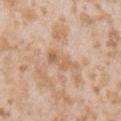This lesion was catalogued during total-body skin photography and was not selected for biopsy. This is a white-light tile. Cropped from a total-body skin-imaging series; the visible field is about 15 mm. Approximately 4 mm at its widest. The subject is a female aged around 25. The lesion is on the arm.